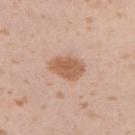Part of a total-body skin-imaging series; this lesion was reviewed on a skin check and was not flagged for biopsy. From the right upper arm. Cropped from a total-body skin-imaging series; the visible field is about 15 mm. A female patient aged approximately 20. Longest diameter approximately 4 mm.